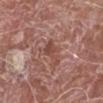Clinical summary: The lesion's longest dimension is about 3.5 mm. Located on the left lower leg. This is a white-light tile. A male patient, aged around 80. A lesion tile, about 15 mm wide, cut from a 3D total-body photograph. Automated tile analysis of the lesion measured a border-irregularity rating of about 6/10, a color-variation rating of about 1.5/10, and a peripheral color-asymmetry measure near 0.5.